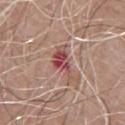biopsy status: total-body-photography surveillance lesion; no biopsy | lighting: white-light illumination | acquisition: total-body-photography crop, ~15 mm field of view | patient: male, aged approximately 80 | body site: the front of the torso | lesion size: ≈4.5 mm.